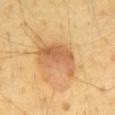Automated tile analysis of the lesion measured an area of roughly 13 mm² and a symmetry-axis asymmetry near 0.45.
Imaged with cross-polarized lighting.
From the mid back.
A roughly 15 mm field-of-view crop from a total-body skin photograph.
A male patient, in their mid-60s.
The recorded lesion diameter is about 5.5 mm.